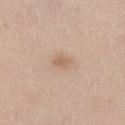follow-up — total-body-photography surveillance lesion; no biopsy
patient — female, aged 38 to 42
illumination — white-light
automated metrics — an average lesion color of about L≈62 a*≈17 b*≈30 (CIELAB), roughly 8 lightness units darker than nearby skin, and a lesion-to-skin contrast of about 6 (normalized; higher = more distinct); an automated nevus-likeness rating near 15 out of 100 and lesion-presence confidence of about 100/100
site — the left lower leg
diameter — ~2.5 mm (longest diameter)
acquisition — total-body-photography crop, ~15 mm field of view